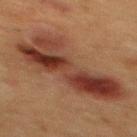Recorded during total-body skin imaging; not selected for excision or biopsy. A male patient, aged 48–52. On the mid back. A close-up tile cropped from a whole-body skin photograph, about 15 mm across.